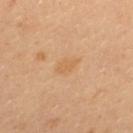Captured during whole-body skin photography for melanoma surveillance; the lesion was not biopsied.
The subject is a female roughly 35 years of age.
Located on the upper back.
Automated image analysis of the tile measured an outline eccentricity of about 0.8 (0 = round, 1 = elongated) and a symmetry-axis asymmetry near 0.25.
A 15 mm close-up tile from a total-body photography series done for melanoma screening.
Captured under cross-polarized illumination.